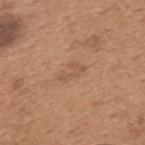No biopsy was performed on this lesion — it was imaged during a full skin examination and was not determined to be concerning.
A male subject roughly 65 years of age.
The lesion is on the upper back.
About 3.5 mm across.
A lesion tile, about 15 mm wide, cut from a 3D total-body photograph.
This is a white-light tile.